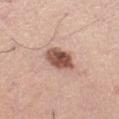Automated image analysis of the tile measured a footprint of about 8.5 mm², a shape eccentricity near 0.6, and two-axis asymmetry of about 0.15. The software also gave a mean CIELAB color near L≈53 a*≈22 b*≈26, about 17 CIELAB-L* units darker than the surrounding skin, and a normalized border contrast of about 11. And it measured border irregularity of about 1.5 on a 0–10 scale and a peripheral color-asymmetry measure near 1.5. It also reported a nevus-likeness score of about 80/100.
On the left thigh.
The subject is a male aged approximately 70.
The tile uses white-light illumination.
A region of skin cropped from a whole-body photographic capture, roughly 15 mm wide.